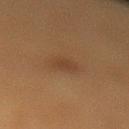illumination=cross-polarized illumination; image source=15 mm crop, total-body photography; lesion size=~2.5 mm (longest diameter); subject=female, aged approximately 40; anatomic site=the left lower leg.